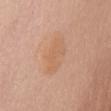Notes:
- follow-up · imaged on a skin check; not biopsied
- automated metrics · a footprint of about 9.5 mm² and a shape-asymmetry score of about 0.2 (0 = symmetric); a border-irregularity index near 3/10 and peripheral color asymmetry of about 1; an automated nevus-likeness rating near 5 out of 100 and lesion-presence confidence of about 100/100
- anatomic site · the chest
- patient · female, aged around 70
- image source · 15 mm crop, total-body photography